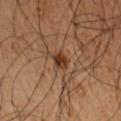  biopsy_status: not biopsied; imaged during a skin examination
  site: upper back
  patient:
    sex: male
    age_approx: 50
  image:
    source: total-body photography crop
    field_of_view_mm: 15